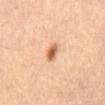Case summary:
* size · ~2.5 mm (longest diameter)
* location · the mid back
* lighting · cross-polarized
* image-analysis metrics · an area of roughly 3.5 mm², a shape eccentricity near 0.75, and a shape-asymmetry score of about 0.15 (0 = symmetric); about 14 CIELAB-L* units darker than the surrounding skin and a normalized lesion–skin contrast near 9; a classifier nevus-likeness of about 100/100 and a detector confidence of about 100 out of 100 that the crop contains a lesion
* acquisition · total-body-photography crop, ~15 mm field of view
* patient · male, about 60 years old